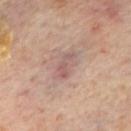- workup · imaged on a skin check; not biopsied
- image-analysis metrics · an area of roughly 3.5 mm², an outline eccentricity of about 0.9 (0 = round, 1 = elongated), and two-axis asymmetry of about 0.45; a mean CIELAB color near L≈53 a*≈19 b*≈21 and a normalized lesion–skin contrast near 6; a border-irregularity rating of about 4.5/10 and radial color variation of about 0; a classifier nevus-likeness of about 0/100 and a detector confidence of about 95 out of 100 that the crop contains a lesion
- image · total-body-photography crop, ~15 mm field of view
- patient · male, about 65 years old
- lesion diameter · ≈3 mm
- illumination · cross-polarized illumination
- location · the mid back